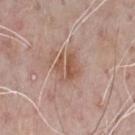- follow-up: catalogued during a skin exam; not biopsied
- anatomic site: the chest
- acquisition: 15 mm crop, total-body photography
- diameter: about 3.5 mm
- subject: male, roughly 70 years of age
- illumination: white-light illumination